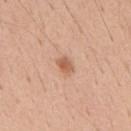Recorded during total-body skin imaging; not selected for excision or biopsy.
The patient is a male aged approximately 40.
The lesion is located on the mid back.
A 15 mm crop from a total-body photograph taken for skin-cancer surveillance.